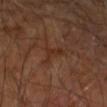follow-up = imaged on a skin check; not biopsied
image source = 15 mm crop, total-body photography
illumination = cross-polarized illumination
automated lesion analysis = a lesion area of about 4 mm², an eccentricity of roughly 0.75, and a symmetry-axis asymmetry near 0.65; a mean CIELAB color near L≈29 a*≈19 b*≈27, a lesion–skin lightness drop of about 5, and a normalized lesion–skin contrast near 6; a lesion-detection confidence of about 95/100
size = ~3 mm (longest diameter)
subject = male, approximately 70 years of age
body site = the arm